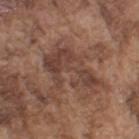The lesion was photographed on a routine skin check and not biopsied; there is no pathology result. A roughly 15 mm field-of-view crop from a total-body skin photograph. A male patient, aged approximately 75. Located on the right upper arm.